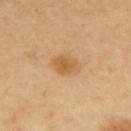workup=imaged on a skin check; not biopsied | tile lighting=cross-polarized | image=~15 mm tile from a whole-body skin photo | TBP lesion metrics=a footprint of about 6.5 mm²; border irregularity of about 1.5 on a 0–10 scale and radial color variation of about 1; an automated nevus-likeness rating near 85 out of 100 and a detector confidence of about 100 out of 100 that the crop contains a lesion | site=the back | subject=female, about 60 years old | lesion size=~3.5 mm (longest diameter).